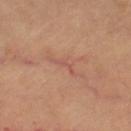biopsy_status: not biopsied; imaged during a skin examination
image:
  source: total-body photography crop
  field_of_view_mm: 15
lighting: cross-polarized
automated_metrics:
  area_mm2_approx: 4.0
  eccentricity: 0.95
  shape_asymmetry: 0.65
  border_irregularity_0_10: 9.0
  color_variation_0_10: 0.0
  peripheral_color_asymmetry: 0.0
  lesion_detection_confidence_0_100: 55
site: left thigh
lesion_size:
  long_diameter_mm_approx: 5.0
patient:
  age_approx: 60